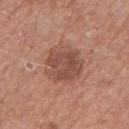{
  "biopsy_status": "not biopsied; imaged during a skin examination",
  "site": "right upper arm",
  "image": {
    "source": "total-body photography crop",
    "field_of_view_mm": 15
  },
  "lighting": "white-light",
  "patient": {
    "sex": "male",
    "age_approx": 55
  },
  "lesion_size": {
    "long_diameter_mm_approx": 5.0
  }
}